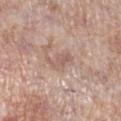<case>
<biopsy_status>not biopsied; imaged during a skin examination</biopsy_status>
<patient>
  <sex>female</sex>
  <age_approx>70</age_approx>
</patient>
<lighting>white-light</lighting>
<automated_metrics>
  <area_mm2_approx>4.5</area_mm2_approx>
  <shape_asymmetry>0.5</shape_asymmetry>
  <border_irregularity_0_10>6.0</border_irregularity_0_10>
  <color_variation_0_10>1.0</color_variation_0_10>
  <peripheral_color_asymmetry>0.0</peripheral_color_asymmetry>
  <nevus_likeness_0_100>0</nevus_likeness_0_100>
</automated_metrics>
<image>
  <source>total-body photography crop</source>
  <field_of_view_mm>15</field_of_view_mm>
</image>
<site>right lower leg</site>
</case>